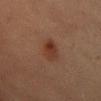notes: no biopsy performed (imaged during a skin exam) | site: the abdomen | image: ~15 mm tile from a whole-body skin photo | subject: female, aged around 65.